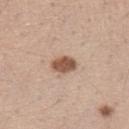follow-up: catalogued during a skin exam; not biopsied | lesion size: ≈3 mm | image: total-body-photography crop, ~15 mm field of view | patient: female, roughly 40 years of age | illumination: white-light | location: the left upper arm.